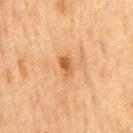<case>
  <biopsy_status>not biopsied; imaged during a skin examination</biopsy_status>
  <patient>
    <sex>male</sex>
    <age_approx>75</age_approx>
  </patient>
  <site>chest</site>
  <image>
    <source>total-body photography crop</source>
    <field_of_view_mm>15</field_of_view_mm>
  </image>
</case>